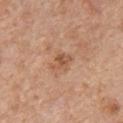- acquisition · 15 mm crop, total-body photography
- body site · the chest
- patient · male, aged around 60
- diameter · ~2.5 mm (longest diameter)
- automated lesion analysis · an average lesion color of about L≈54 a*≈23 b*≈34 (CIELAB) and a normalized lesion–skin contrast near 6.5; a nevus-likeness score of about 5/100 and a detector confidence of about 100 out of 100 that the crop contains a lesion